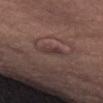The lesion was tiled from a total-body skin photograph and was not biopsied. Captured under white-light illumination. Automated image analysis of the tile measured a shape eccentricity near 0.9 and a symmetry-axis asymmetry near 0.25. And it measured roughly 7 lightness units darker than nearby skin and a lesion-to-skin contrast of about 6.5 (normalized; higher = more distinct). It also reported border irregularity of about 3 on a 0–10 scale, a color-variation rating of about 0/10, and peripheral color asymmetry of about 0. It also reported a classifier nevus-likeness of about 0/100 and a detector confidence of about 65 out of 100 that the crop contains a lesion. The lesion is on the abdomen. Approximately 3 mm at its widest. A region of skin cropped from a whole-body photographic capture, roughly 15 mm wide. A male subject aged around 80.